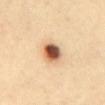Recorded during total-body skin imaging; not selected for excision or biopsy.